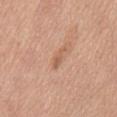- notes · catalogued during a skin exam; not biopsied
- lesion diameter · ≈3 mm
- location · the abdomen
- subject · female, aged around 55
- image source · ~15 mm crop, total-body skin-cancer survey
- lighting · white-light illumination